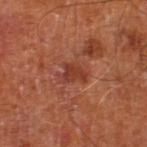workup: imaged on a skin check; not biopsied
diameter: about 3 mm
illumination: cross-polarized illumination
site: the leg
acquisition: total-body-photography crop, ~15 mm field of view
subject: male, aged 63–67